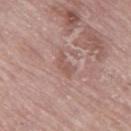Impression: Part of a total-body skin-imaging series; this lesion was reviewed on a skin check and was not flagged for biopsy. Acquisition and patient details: The total-body-photography lesion software estimated a footprint of about 3.5 mm² and a shape eccentricity near 0.9. The analysis additionally found a border-irregularity index near 4.5/10, a color-variation rating of about 1/10, and a peripheral color-asymmetry measure near 0.5. A lesion tile, about 15 mm wide, cut from a 3D total-body photograph. The lesion is on the left thigh. Measured at roughly 3 mm in maximum diameter. A female patient roughly 75 years of age.